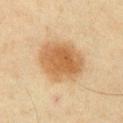Assessment: This lesion was catalogued during total-body skin photography and was not selected for biopsy. Image and clinical context: Longest diameter approximately 5.5 mm. The tile uses cross-polarized illumination. A close-up tile cropped from a whole-body skin photograph, about 15 mm across. The patient is a male about 40 years old. The lesion is on the chest.